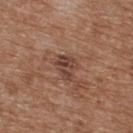follow-up=catalogued during a skin exam; not biopsied
illumination=white-light
size=~3 mm (longest diameter)
subject=female, in their mid- to late 70s
location=the upper back
acquisition=~15 mm crop, total-body skin-cancer survey